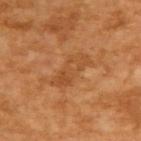workup — total-body-photography surveillance lesion; no biopsy
image source — ~15 mm crop, total-body skin-cancer survey
diameter — about 5 mm
patient — male, approximately 65 years of age
illumination — cross-polarized